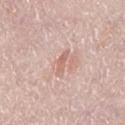notes: imaged on a skin check; not biopsied | anatomic site: the left lower leg | acquisition: total-body-photography crop, ~15 mm field of view | lesion size: ≈2.5 mm | subject: female, aged approximately 40.